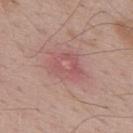notes: total-body-photography surveillance lesion; no biopsy | imaging modality: total-body-photography crop, ~15 mm field of view | subject: male, approximately 70 years of age | location: the upper back | size: ~4.5 mm (longest diameter) | automated lesion analysis: a lesion area of about 11 mm², an outline eccentricity of about 0.6 (0 = round, 1 = elongated), and two-axis asymmetry of about 0.3; a nevus-likeness score of about 0/100 and lesion-presence confidence of about 100/100.